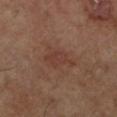– follow-up — total-body-photography surveillance lesion; no biopsy
– anatomic site — the left lower leg
– subject — male, about 65 years old
– image source — 15 mm crop, total-body photography